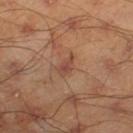biopsy status = total-body-photography surveillance lesion; no biopsy
image = ~15 mm tile from a whole-body skin photo
tile lighting = cross-polarized
subject = male, in their mid-40s
diameter = ~2.5 mm (longest diameter)
site = the right lower leg